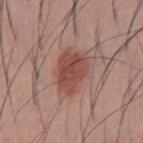This lesion was catalogued during total-body skin photography and was not selected for biopsy. This is a white-light tile. Located on the abdomen. The subject is a male aged 33 to 37. Cropped from a total-body skin-imaging series; the visible field is about 15 mm.